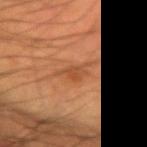Assessment:
No biopsy was performed on this lesion — it was imaged during a full skin examination and was not determined to be concerning.
Clinical summary:
A 15 mm close-up extracted from a 3D total-body photography capture. A male patient in their mid-60s. The lesion is on the right forearm. An algorithmic analysis of the crop reported a mean CIELAB color near L≈45 a*≈24 b*≈35 and about 7 CIELAB-L* units darker than the surrounding skin. The analysis additionally found peripheral color asymmetry of about 1. It also reported a classifier nevus-likeness of about 0/100 and a detector confidence of about 100 out of 100 that the crop contains a lesion.